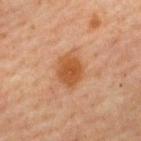workup — total-body-photography surveillance lesion; no biopsy
diameter — ~4 mm (longest diameter)
anatomic site — the upper back
automated lesion analysis — a border-irregularity rating of about 1.5/10 and peripheral color asymmetry of about 1
illumination — cross-polarized
acquisition — total-body-photography crop, ~15 mm field of view
patient — male, aged approximately 60